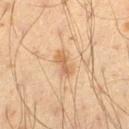Clinical impression: The lesion was tiled from a total-body skin photograph and was not biopsied. Context: Located on the leg. A 15 mm close-up extracted from a 3D total-body photography capture. Captured under cross-polarized illumination. A male patient, approximately 60 years of age.